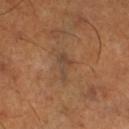Imaged during a routine full-body skin examination; the lesion was not biopsied and no histopathology is available.
A male patient, aged 48 to 52.
This image is a 15 mm lesion crop taken from a total-body photograph.
This is a cross-polarized tile.
The lesion is located on the left lower leg.